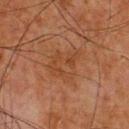follow-up — no biopsy performed (imaged during a skin exam) | automated metrics — a lesion color around L≈35 a*≈20 b*≈29 in CIELAB and a lesion–skin lightness drop of about 5; a border-irregularity rating of about 7/10, a color-variation rating of about 2.5/10, and radial color variation of about 1; a nevus-likeness score of about 0/100 | image — total-body-photography crop, ~15 mm field of view | lesion size — ~5 mm (longest diameter) | illumination — cross-polarized | patient — male, about 65 years old | body site — the upper back.